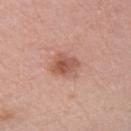notes = catalogued during a skin exam; not biopsied | patient = female, aged approximately 65 | imaging modality = total-body-photography crop, ~15 mm field of view | site = the left upper arm | size = about 3 mm.